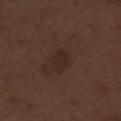This lesion was catalogued during total-body skin photography and was not selected for biopsy. Cropped from a total-body skin-imaging series; the visible field is about 15 mm. A male patient in their 70s. Located on the right lower leg.